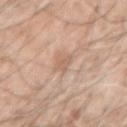Captured during whole-body skin photography for melanoma surveillance; the lesion was not biopsied. From the arm. A male subject, aged approximately 60. Captured under white-light illumination. The total-body-photography lesion software estimated a footprint of about 4 mm² and two-axis asymmetry of about 0.3. The analysis additionally found a within-lesion color-variation index near 1.5/10 and peripheral color asymmetry of about 0.5. The analysis additionally found a classifier nevus-likeness of about 0/100 and a lesion-detection confidence of about 100/100. Measured at roughly 2.5 mm in maximum diameter. A lesion tile, about 15 mm wide, cut from a 3D total-body photograph.